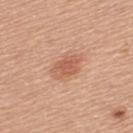* workup: total-body-photography surveillance lesion; no biopsy
* image: total-body-photography crop, ~15 mm field of view
* patient: female, about 55 years old
* automated metrics: a footprint of about 7.5 mm², an eccentricity of roughly 0.8, and a shape-asymmetry score of about 0.25 (0 = symmetric); a border-irregularity index near 3/10, a color-variation rating of about 3/10, and a peripheral color-asymmetry measure near 1; an automated nevus-likeness rating near 85 out of 100 and a detector confidence of about 100 out of 100 that the crop contains a lesion
* lesion size: ~4 mm (longest diameter)
* tile lighting: white-light
* body site: the upper back